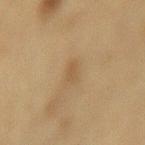Imaged during a routine full-body skin examination; the lesion was not biopsied and no histopathology is available. A female patient about 55 years old. A roughly 15 mm field-of-view crop from a total-body skin photograph. The lesion's longest dimension is about 2 mm. From the mid back. The tile uses cross-polarized illumination.